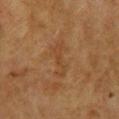Impression:
Part of a total-body skin-imaging series; this lesion was reviewed on a skin check and was not flagged for biopsy.
Image and clinical context:
Imaged with cross-polarized lighting. The recorded lesion diameter is about 4.5 mm. From the head or neck. The total-body-photography lesion software estimated an eccentricity of roughly 0.95 and a shape-asymmetry score of about 0.5 (0 = symmetric). And it measured an automated nevus-likeness rating near 0 out of 100. A female subject, in their 60s. A 15 mm close-up extracted from a 3D total-body photography capture.